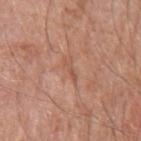The lesion was photographed on a routine skin check and not biopsied; there is no pathology result. The patient is a male approximately 80 years of age. The tile uses white-light illumination. Automated tile analysis of the lesion measured an outline eccentricity of about 0.95 (0 = round, 1 = elongated) and a symmetry-axis asymmetry near 0.4. The software also gave an average lesion color of about L≈54 a*≈24 b*≈31 (CIELAB) and a normalized lesion–skin contrast near 5. And it measured a border-irregularity rating of about 4.5/10, a within-lesion color-variation index near 0/10, and peripheral color asymmetry of about 0. And it measured a nevus-likeness score of about 0/100 and a detector confidence of about 80 out of 100 that the crop contains a lesion. Measured at roughly 2.5 mm in maximum diameter. A 15 mm close-up tile from a total-body photography series done for melanoma screening. The lesion is located on the arm.